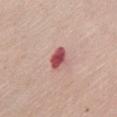A close-up tile cropped from a whole-body skin photograph, about 15 mm across. This is a white-light tile. An algorithmic analysis of the crop reported a footprint of about 4.5 mm², an eccentricity of roughly 0.75, and a symmetry-axis asymmetry near 0.25. It also reported a lesion color around L≈52 a*≈32 b*≈22 in CIELAB, a lesion–skin lightness drop of about 18, and a lesion-to-skin contrast of about 11.5 (normalized; higher = more distinct). And it measured border irregularity of about 2.5 on a 0–10 scale, a within-lesion color-variation index near 3.5/10, and radial color variation of about 1. The analysis additionally found a nevus-likeness score of about 0/100 and lesion-presence confidence of about 100/100. Located on the chest. The subject is a female aged 63–67. The recorded lesion diameter is about 2.5 mm.